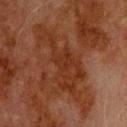Q: Is there a histopathology result?
A: no biopsy performed (imaged during a skin exam)
Q: What is the anatomic site?
A: the upper back
Q: Automated lesion metrics?
A: an area of roughly 20 mm² and an eccentricity of roughly 0.95; internal color variation of about 2 on a 0–10 scale and radial color variation of about 0.5; a lesion-detection confidence of about 90/100
Q: What lighting was used for the tile?
A: cross-polarized illumination
Q: How large is the lesion?
A: ≈10 mm
Q: What is the imaging modality?
A: ~15 mm crop, total-body skin-cancer survey
Q: Patient demographics?
A: male, about 80 years old